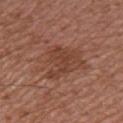Impression:
Imaged during a routine full-body skin examination; the lesion was not biopsied and no histopathology is available.
Background:
A close-up tile cropped from a whole-body skin photograph, about 15 mm across. A female subject aged 58–62. Located on the front of the torso.